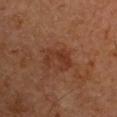Recorded during total-body skin imaging; not selected for excision or biopsy. The lesion-visualizer software estimated a lesion color around L≈33 a*≈23 b*≈29 in CIELAB, roughly 7 lightness units darker than nearby skin, and a normalized lesion–skin contrast near 6.5. The software also gave a nevus-likeness score of about 5/100 and a lesion-detection confidence of about 100/100. The tile uses cross-polarized illumination. Measured at roughly 3.5 mm in maximum diameter. From the right upper arm. A lesion tile, about 15 mm wide, cut from a 3D total-body photograph. A male subject approximately 65 years of age.